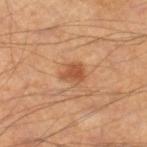Clinical impression: The lesion was tiled from a total-body skin photograph and was not biopsied. Acquisition and patient details: This image is a 15 mm lesion crop taken from a total-body photograph. The subject is a male about 50 years old. The lesion is located on the left leg. Approximately 2.5 mm at its widest. The tile uses cross-polarized illumination. The total-body-photography lesion software estimated a lesion area of about 4.5 mm², a shape eccentricity near 0.55, and a shape-asymmetry score of about 0.3 (0 = symmetric). It also reported a border-irregularity rating of about 3/10 and radial color variation of about 0.5.